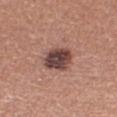{
  "automated_metrics": {
    "area_mm2_approx": 11.0,
    "shape_asymmetry": 0.15
  },
  "lighting": "white-light",
  "site": "leg",
  "image": {
    "source": "total-body photography crop",
    "field_of_view_mm": 15
  },
  "patient": {
    "sex": "female",
    "age_approx": 60
  },
  "lesion_size": {
    "long_diameter_mm_approx": 4.0
  }
}